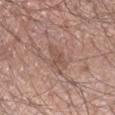The lesion was tiled from a total-body skin photograph and was not biopsied. The lesion is on the right forearm. The subject is a male aged 53 to 57. Imaged with white-light lighting. The lesion-visualizer software estimated a color-variation rating of about 2.5/10 and radial color variation of about 1. And it measured an automated nevus-likeness rating near 0 out of 100 and a lesion-detection confidence of about 100/100. A lesion tile, about 15 mm wide, cut from a 3D total-body photograph.